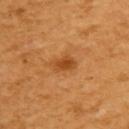No biopsy was performed on this lesion — it was imaged during a full skin examination and was not determined to be concerning. A region of skin cropped from a whole-body photographic capture, roughly 15 mm wide. Longest diameter approximately 3 mm. Imaged with cross-polarized lighting. From the upper back. The patient is a female aged 53 to 57.